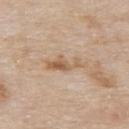image source: 15 mm crop, total-body photography | automated metrics: a normalized border contrast of about 7; a peripheral color-asymmetry measure near 1 | patient: male, approximately 85 years of age or older | site: the back.